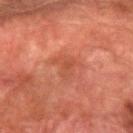Impression:
This lesion was catalogued during total-body skin photography and was not selected for biopsy.
Acquisition and patient details:
Longest diameter approximately 3.5 mm. Cropped from a whole-body photographic skin survey; the tile spans about 15 mm. The lesion is on the arm. A male subject, aged 78 to 82. Captured under cross-polarized illumination.